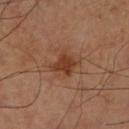Recorded during total-body skin imaging; not selected for excision or biopsy. Located on the right lower leg. A 15 mm crop from a total-body photograph taken for skin-cancer surveillance. A male patient approximately 60 years of age. Captured under cross-polarized illumination. Approximately 3.5 mm at its widest.